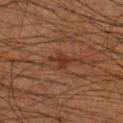Part of a total-body skin-imaging series; this lesion was reviewed on a skin check and was not flagged for biopsy. On the right lower leg. A male subject, in their mid-50s. A 15 mm close-up tile from a total-body photography series done for melanoma screening. The lesion-visualizer software estimated a lesion area of about 4 mm².